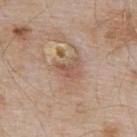{"biopsy_status": "not biopsied; imaged during a skin examination"}